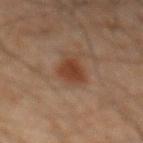Imaged during a routine full-body skin examination; the lesion was not biopsied and no histopathology is available. The recorded lesion diameter is about 3 mm. A male subject, roughly 65 years of age. Captured under cross-polarized illumination. From the abdomen. Cropped from a whole-body photographic skin survey; the tile spans about 15 mm. Automated tile analysis of the lesion measured a border-irregularity index near 2.5/10 and a within-lesion color-variation index near 1.5/10.